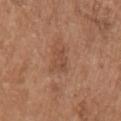Imaged during a routine full-body skin examination; the lesion was not biopsied and no histopathology is available.
Captured under white-light illumination.
Located on the left upper arm.
A male subject aged approximately 75.
A 15 mm close-up extracted from a 3D total-body photography capture.